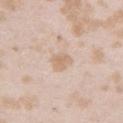Q: Was this lesion biopsied?
A: total-body-photography surveillance lesion; no biopsy
Q: Who is the patient?
A: female, approximately 25 years of age
Q: Automated lesion metrics?
A: an average lesion color of about L≈68 a*≈15 b*≈30 (CIELAB) and a lesion-to-skin contrast of about 6 (normalized; higher = more distinct)
Q: How was this image acquired?
A: ~15 mm tile from a whole-body skin photo
Q: What is the anatomic site?
A: the left upper arm
Q: How large is the lesion?
A: ≈2.5 mm